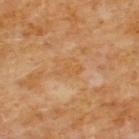The lesion was photographed on a routine skin check and not biopsied; there is no pathology result.
A roughly 15 mm field-of-view crop from a total-body skin photograph.
The subject is a male in their 60s.
The tile uses cross-polarized illumination.
Approximately 3 mm at its widest.
The total-body-photography lesion software estimated a lesion area of about 3 mm², an outline eccentricity of about 0.9 (0 = round, 1 = elongated), and a symmetry-axis asymmetry near 0.5. The software also gave roughly 5 lightness units darker than nearby skin and a lesion-to-skin contrast of about 5 (normalized; higher = more distinct). It also reported internal color variation of about 0.5 on a 0–10 scale and a peripheral color-asymmetry measure near 0. The analysis additionally found an automated nevus-likeness rating near 0 out of 100 and lesion-presence confidence of about 100/100.
On the chest.